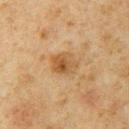Q: Was a biopsy performed?
A: imaged on a skin check; not biopsied
Q: Where on the body is the lesion?
A: the left upper arm
Q: How was this image acquired?
A: 15 mm crop, total-body photography
Q: What are the patient's age and sex?
A: male, roughly 60 years of age
Q: Lesion size?
A: about 3 mm